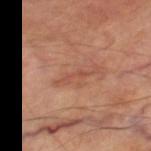biopsy status: catalogued during a skin exam; not biopsied
image-analysis metrics: a lesion color around L≈49 a*≈25 b*≈29 in CIELAB, a lesion–skin lightness drop of about 7, and a lesion-to-skin contrast of about 5 (normalized; higher = more distinct); an automated nevus-likeness rating near 0 out of 100 and a lesion-detection confidence of about 80/100
subject: male, in their 70s
image source: ~15 mm crop, total-body skin-cancer survey
lesion diameter: ~3 mm (longest diameter)
anatomic site: the right thigh